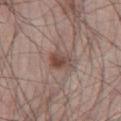{"biopsy_status": "not biopsied; imaged during a skin examination", "automated_metrics": {"area_mm2_approx": 6.5, "eccentricity": 0.65, "shape_asymmetry": 0.25, "cielab_L": 47, "cielab_a": 17, "cielab_b": 23, "vs_skin_darker_L": 10.0, "vs_skin_contrast_norm": 7.5}, "lesion_size": {"long_diameter_mm_approx": 3.5}, "patient": {"sex": "male", "age_approx": 55}, "site": "right thigh", "image": {"source": "total-body photography crop", "field_of_view_mm": 15}, "lighting": "white-light"}